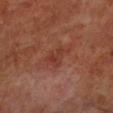Case summary:
- workup — total-body-photography surveillance lesion; no biopsy
- acquisition — ~15 mm crop, total-body skin-cancer survey
- subject — male, in their 70s
- site — the left lower leg
- illumination — cross-polarized illumination
- size — about 3 mm